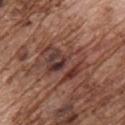{"biopsy_status": "not biopsied; imaged during a skin examination", "image": {"source": "total-body photography crop", "field_of_view_mm": 15}, "patient": {"sex": "male", "age_approx": 75}, "site": "chest"}